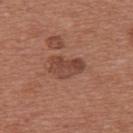Notes:
• acquisition: total-body-photography crop, ~15 mm field of view
• anatomic site: the back
• patient: female, aged approximately 50